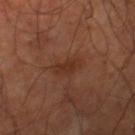Imaged during a routine full-body skin examination; the lesion was not biopsied and no histopathology is available.
This is a cross-polarized tile.
Automated image analysis of the tile measured an automated nevus-likeness rating near 0 out of 100 and lesion-presence confidence of about 100/100.
Located on the right lower leg.
The subject is a male aged 58 to 62.
Measured at roughly 3.5 mm in maximum diameter.
A roughly 15 mm field-of-view crop from a total-body skin photograph.